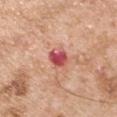{
  "patient": {
    "sex": "male",
    "age_approx": 55
  },
  "lighting": "white-light",
  "image": {
    "source": "total-body photography crop",
    "field_of_view_mm": 15
  },
  "lesion_size": {
    "long_diameter_mm_approx": 3.0
  },
  "site": "left upper arm"
}